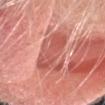| field | value |
|---|---|
| workup | no biopsy performed (imaged during a skin exam) |